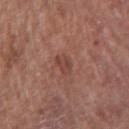Assessment: Captured during whole-body skin photography for melanoma surveillance; the lesion was not biopsied. Acquisition and patient details: From the right upper arm. This image is a 15 mm lesion crop taken from a total-body photograph. Measured at roughly 3 mm in maximum diameter. Imaged with white-light lighting. A male subject aged 63 to 67.